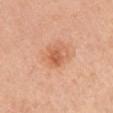| feature | finding |
|---|---|
| follow-up | total-body-photography surveillance lesion; no biopsy |
| size | about 2.5 mm |
| image source | 15 mm crop, total-body photography |
| illumination | white-light illumination |
| image-analysis metrics | a shape eccentricity near 0.65; a lesion color around L≈59 a*≈28 b*≈37 in CIELAB, a lesion–skin lightness drop of about 10, and a lesion-to-skin contrast of about 6.5 (normalized; higher = more distinct) |
| patient | female, aged around 45 |
| body site | the arm |